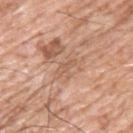This lesion was catalogued during total-body skin photography and was not selected for biopsy. A male subject, about 60 years old. Approximately 3 mm at its widest. Cropped from a whole-body photographic skin survey; the tile spans about 15 mm. Captured under white-light illumination. On the upper back. The lesion-visualizer software estimated a footprint of about 2.5 mm², a shape eccentricity near 0.95, and a symmetry-axis asymmetry near 0.35. And it measured a lesion color around L≈58 a*≈21 b*≈32 in CIELAB, a lesion–skin lightness drop of about 7, and a normalized border contrast of about 5. And it measured a border-irregularity rating of about 4/10 and radial color variation of about 0.